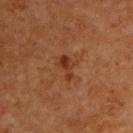Q: Was this lesion biopsied?
A: total-body-photography surveillance lesion; no biopsy
Q: How was this image acquired?
A: 15 mm crop, total-body photography
Q: Lesion location?
A: the upper back
Q: Automated lesion metrics?
A: a shape-asymmetry score of about 0.5 (0 = symmetric); a within-lesion color-variation index near 0.5/10 and radial color variation of about 0; a classifier nevus-likeness of about 0/100 and a lesion-detection confidence of about 100/100
Q: Who is the patient?
A: male, roughly 65 years of age